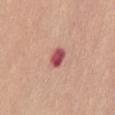biopsy status: total-body-photography surveillance lesion; no biopsy | acquisition: total-body-photography crop, ~15 mm field of view | tile lighting: white-light | anatomic site: the front of the torso | subject: female, approximately 50 years of age | lesion size: ~2.5 mm (longest diameter) | automated metrics: an average lesion color of about L≈52 a*≈34 b*≈23 (CIELAB), roughly 16 lightness units darker than nearby skin, and a lesion-to-skin contrast of about 11 (normalized; higher = more distinct); a border-irregularity rating of about 1.5/10, a color-variation rating of about 4.5/10, and peripheral color asymmetry of about 1.5.